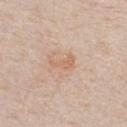follow-up = catalogued during a skin exam; not biopsied
patient = male, in their 30s
automated metrics = a mean CIELAB color near L≈64 a*≈20 b*≈31, roughly 7 lightness units darker than nearby skin, and a normalized lesion–skin contrast near 5; a border-irregularity index near 5/10
body site = the chest
lesion diameter = ≈3 mm
image = ~15 mm tile from a whole-body skin photo